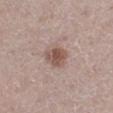Q: Is there a histopathology result?
A: no biopsy performed (imaged during a skin exam)
Q: What kind of image is this?
A: ~15 mm crop, total-body skin-cancer survey
Q: Illumination type?
A: white-light
Q: What is the lesion's diameter?
A: ≈3 mm
Q: Lesion location?
A: the leg
Q: What are the patient's age and sex?
A: female, aged 38 to 42